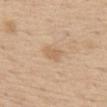Q: Was this lesion biopsied?
A: catalogued during a skin exam; not biopsied
Q: Lesion location?
A: the front of the torso
Q: Automated lesion metrics?
A: an eccentricity of roughly 0.8 and a symmetry-axis asymmetry near 0.25; an average lesion color of about L≈63 a*≈16 b*≈34 (CIELAB), roughly 7 lightness units darker than nearby skin, and a lesion-to-skin contrast of about 5 (normalized; higher = more distinct); a border-irregularity index near 2.5/10 and a within-lesion color-variation index near 1/10; a classifier nevus-likeness of about 0/100 and a detector confidence of about 100 out of 100 that the crop contains a lesion
Q: What kind of image is this?
A: ~15 mm tile from a whole-body skin photo
Q: How large is the lesion?
A: about 3 mm
Q: Patient demographics?
A: male, approximately 70 years of age